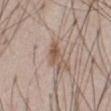notes=catalogued during a skin exam; not biopsied
site=the abdomen
subject=male, aged approximately 60
imaging modality=15 mm crop, total-body photography
lesion diameter=≈3.5 mm
automated lesion analysis=a lesion area of about 5 mm², an eccentricity of roughly 0.85, and two-axis asymmetry of about 0.35